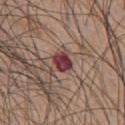Findings:
• biopsy status — total-body-photography surveillance lesion; no biopsy
• patient — male, aged approximately 45
• lighting — white-light illumination
• body site — the front of the torso
• acquisition — total-body-photography crop, ~15 mm field of view